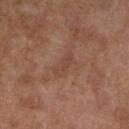The lesion was photographed on a routine skin check and not biopsied; there is no pathology result. This is a cross-polarized tile. The total-body-photography lesion software estimated a within-lesion color-variation index near 1/10 and peripheral color asymmetry of about 0.5. It also reported a nevus-likeness score of about 0/100 and a lesion-detection confidence of about 95/100. On the leg. A female subject aged approximately 60. Cropped from a total-body skin-imaging series; the visible field is about 15 mm.